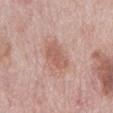This lesion was catalogued during total-body skin photography and was not selected for biopsy. The lesion-visualizer software estimated a lesion area of about 7 mm², an outline eccentricity of about 0.85 (0 = round, 1 = elongated), and a symmetry-axis asymmetry near 0.25. The analysis additionally found an average lesion color of about L≈58 a*≈23 b*≈26 (CIELAB), roughly 9 lightness units darker than nearby skin, and a normalized lesion–skin contrast near 6. And it measured a border-irregularity rating of about 2.5/10, a within-lesion color-variation index near 2/10, and radial color variation of about 0.5. The analysis additionally found an automated nevus-likeness rating near 50 out of 100 and lesion-presence confidence of about 100/100. A male patient aged approximately 75. Approximately 4 mm at its widest. Cropped from a total-body skin-imaging series; the visible field is about 15 mm. The lesion is located on the mid back.